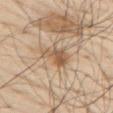Captured during whole-body skin photography for melanoma surveillance; the lesion was not biopsied.
A 15 mm close-up tile from a total-body photography series done for melanoma screening.
A male patient, approximately 80 years of age.
An algorithmic analysis of the crop reported a lesion color around L≈57 a*≈17 b*≈33 in CIELAB, roughly 11 lightness units darker than nearby skin, and a normalized lesion–skin contrast near 7.5. The software also gave a lesion-detection confidence of about 100/100.
From the back.
Captured under white-light illumination.
Longest diameter approximately 4 mm.